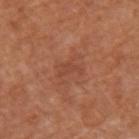Q: Was this lesion biopsied?
A: no biopsy performed (imaged during a skin exam)
Q: What are the patient's age and sex?
A: female, aged around 65
Q: What is the anatomic site?
A: the left arm
Q: Illumination type?
A: white-light illumination
Q: Lesion size?
A: about 3 mm
Q: What is the imaging modality?
A: 15 mm crop, total-body photography